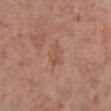workup=total-body-photography surveillance lesion; no biopsy | diameter=about 3 mm | illumination=white-light | imaging modality=15 mm crop, total-body photography | subject=male, aged 68–72 | anatomic site=the leg.